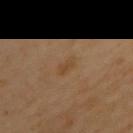Clinical summary: On the back. A 15 mm close-up tile from a total-body photography series done for melanoma screening. The lesion-visualizer software estimated a lesion area of about 3 mm², an outline eccentricity of about 0.85 (0 = round, 1 = elongated), and a symmetry-axis asymmetry near 0.35. And it measured a mean CIELAB color near L≈38 a*≈14 b*≈30, roughly 4 lightness units darker than nearby skin, and a lesion-to-skin contrast of about 5 (normalized; higher = more distinct). The analysis additionally found a detector confidence of about 100 out of 100 that the crop contains a lesion. A female subject aged around 60. Imaged with cross-polarized lighting. The recorded lesion diameter is about 2.5 mm.